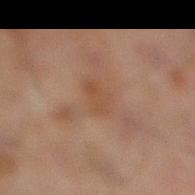Part of a total-body skin-imaging series; this lesion was reviewed on a skin check and was not flagged for biopsy. A male subject in their mid-40s. Captured under cross-polarized illumination. Approximately 3 mm at its widest. A 15 mm crop from a total-body photograph taken for skin-cancer surveillance. Located on the leg.